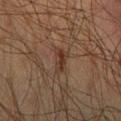Part of a total-body skin-imaging series; this lesion was reviewed on a skin check and was not flagged for biopsy. A male patient aged around 35. The tile uses cross-polarized illumination. A 15 mm close-up tile from a total-body photography series done for melanoma screening. From the back. Measured at roughly 3 mm in maximum diameter.